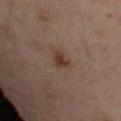workup: no biopsy performed (imaged during a skin exam)
subject: female, aged approximately 55
TBP lesion metrics: a footprint of about 4 mm², an outline eccentricity of about 0.65 (0 = round, 1 = elongated), and two-axis asymmetry of about 0.3; an automated nevus-likeness rating near 85 out of 100 and a detector confidence of about 100 out of 100 that the crop contains a lesion
image source: ~15 mm tile from a whole-body skin photo
body site: the arm
lesion size: ~2.5 mm (longest diameter)